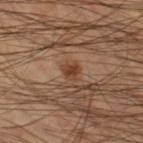Assessment:
This lesion was catalogued during total-body skin photography and was not selected for biopsy.
Image and clinical context:
From the left thigh. An algorithmic analysis of the crop reported a footprint of about 3.5 mm² and an eccentricity of roughly 0.3. The software also gave an average lesion color of about L≈41 a*≈20 b*≈31 (CIELAB), roughly 8 lightness units darker than nearby skin, and a normalized lesion–skin contrast near 7.5. It also reported a border-irregularity rating of about 2/10, internal color variation of about 3 on a 0–10 scale, and peripheral color asymmetry of about 1. This is a cross-polarized tile. A 15 mm close-up tile from a total-body photography series done for melanoma screening. A male subject aged 48–52. Measured at roughly 2 mm in maximum diameter.